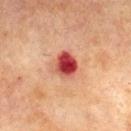<record>
<biopsy_status>not biopsied; imaged during a skin examination</biopsy_status>
<patient>
  <sex>male</sex>
  <age_approx>65</age_approx>
</patient>
<lesion_size>
  <long_diameter_mm_approx>3.0</long_diameter_mm_approx>
</lesion_size>
<lighting>cross-polarized</lighting>
<site>mid back</site>
<image>
  <source>total-body photography crop</source>
  <field_of_view_mm>15</field_of_view_mm>
</image>
</record>